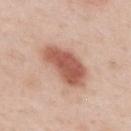<case>
  <lesion_size>
    <long_diameter_mm_approx>6.0</long_diameter_mm_approx>
  </lesion_size>
  <lighting>white-light</lighting>
  <patient>
    <sex>male</sex>
    <age_approx>50</age_approx>
  </patient>
  <site>upper back</site>
  <image>
    <source>total-body photography crop</source>
    <field_of_view_mm>15</field_of_view_mm>
  </image>
</case>